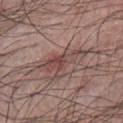Part of a total-body skin-imaging series; this lesion was reviewed on a skin check and was not flagged for biopsy.
The tile uses white-light illumination.
A male patient roughly 40 years of age.
Located on the chest.
Automated image analysis of the tile measured a lesion area of about 8.5 mm², an eccentricity of roughly 0.95, and a shape-asymmetry score of about 0.4 (0 = symmetric). The analysis additionally found border irregularity of about 6.5 on a 0–10 scale, a within-lesion color-variation index near 5/10, and a peripheral color-asymmetry measure near 1.5.
A close-up tile cropped from a whole-body skin photograph, about 15 mm across.
The recorded lesion diameter is about 6 mm.